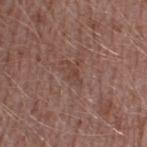Part of a total-body skin-imaging series; this lesion was reviewed on a skin check and was not flagged for biopsy. On the right thigh. The lesion's longest dimension is about 2.5 mm. A lesion tile, about 15 mm wide, cut from a 3D total-body photograph. Captured under white-light illumination. Automated image analysis of the tile measured a footprint of about 2 mm² and a shape-asymmetry score of about 0.5 (0 = symmetric). It also reported a nevus-likeness score of about 0/100 and lesion-presence confidence of about 95/100. A male patient aged 43–47.